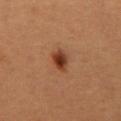{
  "biopsy_status": "not biopsied; imaged during a skin examination",
  "image": {
    "source": "total-body photography crop",
    "field_of_view_mm": 15
  },
  "lighting": "cross-polarized",
  "patient": {
    "sex": "male",
    "age_approx": 50
  },
  "site": "abdomen",
  "automated_metrics": {
    "vs_skin_contrast_norm": 10.0,
    "border_irregularity_0_10": 2.0,
    "color_variation_0_10": 4.5,
    "peripheral_color_asymmetry": 1.5,
    "nevus_likeness_0_100": 100,
    "lesion_detection_confidence_0_100": 100
  }
}